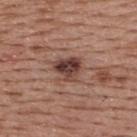Imaged during a routine full-body skin examination; the lesion was not biopsied and no histopathology is available. A male subject aged 53 to 57. The lesion's longest dimension is about 3 mm. Imaged with white-light lighting. The lesion is on the upper back. A close-up tile cropped from a whole-body skin photograph, about 15 mm across.